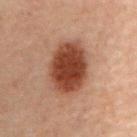workup — no biopsy performed (imaged during a skin exam) | body site — the back | patient — male, approximately 70 years of age | illumination — cross-polarized | size — ~6.5 mm (longest diameter) | imaging modality — ~15 mm crop, total-body skin-cancer survey.